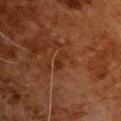<lesion>
  <biopsy_status>not biopsied; imaged during a skin examination</biopsy_status>
  <site>chest</site>
  <lesion_size>
    <long_diameter_mm_approx>3.0</long_diameter_mm_approx>
  </lesion_size>
  <automated_metrics>
    <area_mm2_approx>3.0</area_mm2_approx>
  </automated_metrics>
  <image>
    <source>total-body photography crop</source>
    <field_of_view_mm>15</field_of_view_mm>
  </image>
  <lighting>cross-polarized</lighting>
  <patient>
    <sex>male</sex>
    <age_approx>80</age_approx>
  </patient>
</lesion>